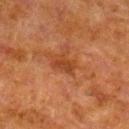Acquisition and patient details: A 15 mm close-up extracted from a 3D total-body photography capture. The lesion's longest dimension is about 2.5 mm. On the leg. Automated tile analysis of the lesion measured an area of roughly 4 mm². It also reported an average lesion color of about L≈34 a*≈23 b*≈32 (CIELAB), a lesion–skin lightness drop of about 7, and a normalized lesion–skin contrast near 6.5. It also reported a nevus-likeness score of about 0/100 and a detector confidence of about 100 out of 100 that the crop contains a lesion. The tile uses cross-polarized illumination. A male patient, roughly 80 years of age.